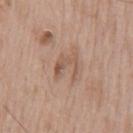Imaged during a routine full-body skin examination; the lesion was not biopsied and no histopathology is available.
Captured under white-light illumination.
On the front of the torso.
A roughly 15 mm field-of-view crop from a total-body skin photograph.
Longest diameter approximately 2.5 mm.
A male patient, aged approximately 65.